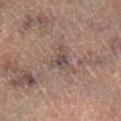* follow-up — imaged on a skin check; not biopsied
* subject — male, aged 63 to 67
* size — ~2.5 mm (longest diameter)
* anatomic site — the leg
* tile lighting — cross-polarized illumination
* image source — ~15 mm tile from a whole-body skin photo